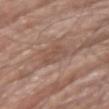Imaged during a routine full-body skin examination; the lesion was not biopsied and no histopathology is available.
A roughly 15 mm field-of-view crop from a total-body skin photograph.
Automated tile analysis of the lesion measured internal color variation of about 2 on a 0–10 scale and a peripheral color-asymmetry measure near 0.5. And it measured a detector confidence of about 95 out of 100 that the crop contains a lesion.
From the left upper arm.
The recorded lesion diameter is about 3.5 mm.
A male patient approximately 65 years of age.
Captured under white-light illumination.